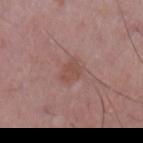Captured during whole-body skin photography for melanoma surveillance; the lesion was not biopsied. The lesion is located on the arm. A male subject aged 48 to 52. A 15 mm crop from a total-body photograph taken for skin-cancer surveillance.